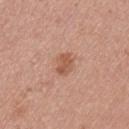This lesion was catalogued during total-body skin photography and was not selected for biopsy. The lesion's longest dimension is about 2.5 mm. Cropped from a whole-body photographic skin survey; the tile spans about 15 mm. Imaged with white-light lighting. A male subject aged approximately 25. From the chest.